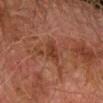notes: total-body-photography surveillance lesion; no biopsy.